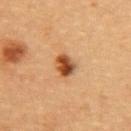  automated_metrics:
    area_mm2_approx: 5.5
    eccentricity: 0.6
    shape_asymmetry: 0.15
    nevus_likeness_0_100: 100
  lighting: cross-polarized
  image:
    source: total-body photography crop
    field_of_view_mm: 15
  patient:
    sex: female
    age_approx: 30
  site: upper back
  lesion_size:
    long_diameter_mm_approx: 3.0